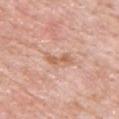notes — catalogued during a skin exam; not biopsied | patient — male, in their mid- to late 50s | site — the chest | acquisition — 15 mm crop, total-body photography | diameter — ~3.5 mm (longest diameter) | tile lighting — white-light.